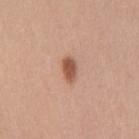Clinical impression:
Recorded during total-body skin imaging; not selected for excision or biopsy.